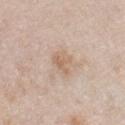Clinical impression: Recorded during total-body skin imaging; not selected for excision or biopsy. Image and clinical context: From the chest. Imaged with white-light lighting. The subject is a male in their 40s. Measured at roughly 3 mm in maximum diameter. Cropped from a whole-body photographic skin survey; the tile spans about 15 mm. The total-body-photography lesion software estimated border irregularity of about 3 on a 0–10 scale, a within-lesion color-variation index near 3/10, and peripheral color asymmetry of about 1. The analysis additionally found a nevus-likeness score of about 0/100 and a lesion-detection confidence of about 100/100.